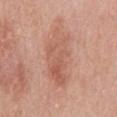Impression:
The lesion was photographed on a routine skin check and not biopsied; there is no pathology result.
Background:
A roughly 15 mm field-of-view crop from a total-body skin photograph. A female subject, aged 38 to 42. From the back. Automated image analysis of the tile measured a footprint of about 18 mm². The analysis additionally found lesion-presence confidence of about 100/100. The lesion's longest dimension is about 8 mm.